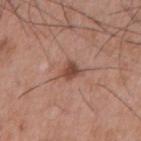notes: catalogued during a skin exam; not biopsied
automated metrics: a footprint of about 4.5 mm², a shape eccentricity near 0.55, and a shape-asymmetry score of about 0.35 (0 = symmetric); a lesion–skin lightness drop of about 11; an automated nevus-likeness rating near 85 out of 100 and a detector confidence of about 100 out of 100 that the crop contains a lesion
lesion diameter: ~3 mm (longest diameter)
image: 15 mm crop, total-body photography
subject: male, aged approximately 55
site: the front of the torso
illumination: white-light